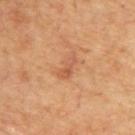Recorded during total-body skin imaging; not selected for excision or biopsy. Approximately 3.5 mm at its widest. The lesion is on the upper back. The patient is roughly 65 years of age. The tile uses cross-polarized illumination. A 15 mm close-up tile from a total-body photography series done for melanoma screening.